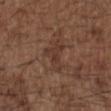{
  "biopsy_status": "not biopsied; imaged during a skin examination",
  "patient": {
    "sex": "male",
    "age_approx": 50
  },
  "lesion_size": {
    "long_diameter_mm_approx": 3.5
  },
  "site": "upper back",
  "image": {
    "source": "total-body photography crop",
    "field_of_view_mm": 15
  },
  "lighting": "white-light"
}